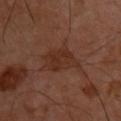Imaged during a routine full-body skin examination; the lesion was not biopsied and no histopathology is available. A close-up tile cropped from a whole-body skin photograph, about 15 mm across. Located on the back. A male subject aged 48–52. Imaged with cross-polarized lighting. Measured at roughly 4.5 mm in maximum diameter.